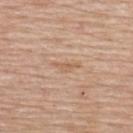* biopsy status — imaged on a skin check; not biopsied